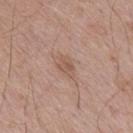* workup — imaged on a skin check; not biopsied
* automated lesion analysis — a nevus-likeness score of about 5/100
* image source — total-body-photography crop, ~15 mm field of view
* subject — male, approximately 70 years of age
* body site — the mid back